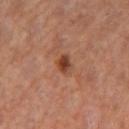No biopsy was performed on this lesion — it was imaged during a full skin examination and was not determined to be concerning. The patient is a female approximately 55 years of age. The lesion is on the right thigh. A lesion tile, about 15 mm wide, cut from a 3D total-body photograph. The lesion-visualizer software estimated an area of roughly 3.5 mm². And it measured border irregularity of about 2 on a 0–10 scale and radial color variation of about 1.5. The analysis additionally found an automated nevus-likeness rating near 90 out of 100 and a lesion-detection confidence of about 100/100.